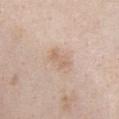follow-up: catalogued during a skin exam; not biopsied
subject: female, in their mid- to late 70s
illumination: white-light illumination
location: the chest
image source: 15 mm crop, total-body photography